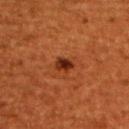notes = no biopsy performed (imaged during a skin exam)
subject = male, roughly 45 years of age
image source = total-body-photography crop, ~15 mm field of view
body site = the upper back